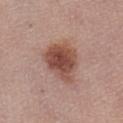Impression:
Captured during whole-body skin photography for melanoma surveillance; the lesion was not biopsied.
Acquisition and patient details:
A female patient roughly 55 years of age. The lesion is located on the abdomen. A roughly 15 mm field-of-view crop from a total-body skin photograph. About 5.5 mm across. Imaged with white-light lighting.